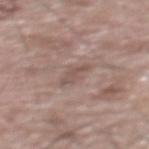The lesion is located on the upper back.
A lesion tile, about 15 mm wide, cut from a 3D total-body photograph.
Imaged with white-light lighting.
Automated tile analysis of the lesion measured a footprint of about 2.5 mm² and a symmetry-axis asymmetry near 0.5.
The patient is a male approximately 70 years of age.